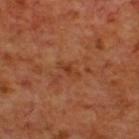biopsy status=total-body-photography surveillance lesion; no biopsy
tile lighting=cross-polarized
patient=male, about 70 years old
location=the upper back
size=≈2.5 mm
image source=total-body-photography crop, ~15 mm field of view